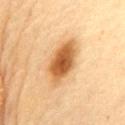follow-up: total-body-photography surveillance lesion; no biopsy
imaging modality: 15 mm crop, total-body photography
diameter: about 6.5 mm
subject: female, aged 78 to 82
illumination: cross-polarized
body site: the mid back